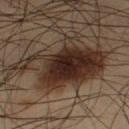Part of a total-body skin-imaging series; this lesion was reviewed on a skin check and was not flagged for biopsy.
The patient is a male in their mid-30s.
Captured under cross-polarized illumination.
The total-body-photography lesion software estimated an average lesion color of about L≈26 a*≈13 b*≈20 (CIELAB) and a normalized lesion–skin contrast near 12.5. The software also gave peripheral color asymmetry of about 2.5. It also reported a classifier nevus-likeness of about 100/100 and a detector confidence of about 100 out of 100 that the crop contains a lesion.
Measured at roughly 10 mm in maximum diameter.
A 15 mm close-up extracted from a 3D total-body photography capture.
Located on the left thigh.